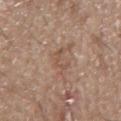Assessment: This lesion was catalogued during total-body skin photography and was not selected for biopsy. Context: Cropped from a total-body skin-imaging series; the visible field is about 15 mm. The lesion is on the back. The tile uses white-light illumination. The lesion's longest dimension is about 3.5 mm. A male patient, about 65 years old.